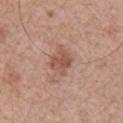No biopsy was performed on this lesion — it was imaged during a full skin examination and was not determined to be concerning. About 3 mm across. The patient is a male aged around 65. A lesion tile, about 15 mm wide, cut from a 3D total-body photograph. Located on the chest.